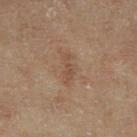Q: Was a biopsy performed?
A: imaged on a skin check; not biopsied
Q: Who is the patient?
A: male, in their 70s
Q: What is the lesion's diameter?
A: about 3.5 mm
Q: What did automated image analysis measure?
A: a within-lesion color-variation index near 0.5/10 and a peripheral color-asymmetry measure near 0; a detector confidence of about 100 out of 100 that the crop contains a lesion
Q: Where on the body is the lesion?
A: the leg
Q: How was this image acquired?
A: ~15 mm tile from a whole-body skin photo